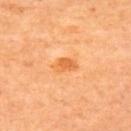{
  "site": "upper back",
  "image": {
    "source": "total-body photography crop",
    "field_of_view_mm": 15
  },
  "patient": {
    "sex": "female",
    "age_approx": 65
  }
}